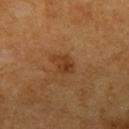workup — imaged on a skin check; not biopsied
lighting — cross-polarized
image source — ~15 mm tile from a whole-body skin photo
size — ≈3 mm
subject — male, aged 58 to 62
site — the left upper arm